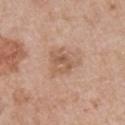| key | value |
|---|---|
| workup | no biopsy performed (imaged during a skin exam) |
| lighting | white-light illumination |
| size | ≈4 mm |
| location | the chest |
| imaging modality | ~15 mm crop, total-body skin-cancer survey |
| patient | male, roughly 65 years of age |